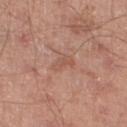Q: Was this lesion biopsied?
A: total-body-photography surveillance lesion; no biopsy
Q: What is the imaging modality?
A: ~15 mm tile from a whole-body skin photo
Q: What lighting was used for the tile?
A: white-light
Q: Automated lesion metrics?
A: a classifier nevus-likeness of about 0/100 and lesion-presence confidence of about 100/100
Q: Patient demographics?
A: male, in their mid- to late 50s
Q: Lesion location?
A: the right lower leg